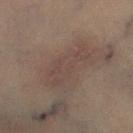notes — no biopsy performed (imaged during a skin exam) | subject — male, aged approximately 55 | image-analysis metrics — a border-irregularity index near 4/10, a within-lesion color-variation index near 2/10, and radial color variation of about 1; an automated nevus-likeness rating near 0 out of 100 and lesion-presence confidence of about 70/100 | image — total-body-photography crop, ~15 mm field of view | tile lighting — cross-polarized illumination | anatomic site — the right lower leg | diameter — ~6 mm (longest diameter).